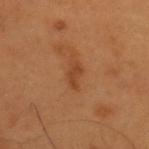{
  "biopsy_status": "not biopsied; imaged during a skin examination",
  "site": "back",
  "automated_metrics": {
    "area_mm2_approx": 3.5,
    "eccentricity": 0.85,
    "shape_asymmetry": 0.35,
    "vs_skin_contrast_norm": 6.5,
    "nevus_likeness_0_100": 0,
    "lesion_detection_confidence_0_100": 100
  },
  "lesion_size": {
    "long_diameter_mm_approx": 3.0
  },
  "image": {
    "source": "total-body photography crop",
    "field_of_view_mm": 15
  },
  "patient": {
    "sex": "male",
    "age_approx": 55
  }
}